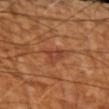Part of a total-body skin-imaging series; this lesion was reviewed on a skin check and was not flagged for biopsy. Longest diameter approximately 2.5 mm. From the arm. This is a cross-polarized tile. A 15 mm crop from a total-body photograph taken for skin-cancer surveillance. The subject is about 65 years old.